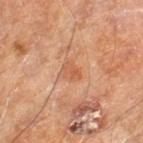Clinical summary:
The patient is a male aged 58–62. Cropped from a whole-body photographic skin survey; the tile spans about 15 mm. The lesion is located on the right leg.